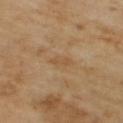Recorded during total-body skin imaging; not selected for excision or biopsy. A 15 mm close-up extracted from a 3D total-body photography capture. The subject is a male in their mid-60s.